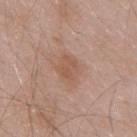Case summary:
– follow-up: imaged on a skin check; not biopsied
– location: the mid back
– imaging modality: 15 mm crop, total-body photography
– subject: male, aged 53–57